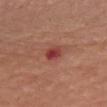The lesion was tiled from a total-body skin photograph and was not biopsied.
The subject is a female about 65 years old.
A close-up tile cropped from a whole-body skin photograph, about 15 mm across.
On the chest.